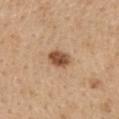notes: imaged on a skin check; not biopsied | automated metrics: a footprint of about 5 mm², an outline eccentricity of about 0.75 (0 = round, 1 = elongated), and a symmetry-axis asymmetry near 0.15; a within-lesion color-variation index near 3.5/10; a classifier nevus-likeness of about 90/100 and a lesion-detection confidence of about 100/100 | image: total-body-photography crop, ~15 mm field of view | lesion size: ≈3 mm | body site: the upper back | lighting: white-light | subject: female, aged approximately 60.